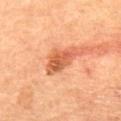Q: Was this lesion biopsied?
A: total-body-photography surveillance lesion; no biopsy